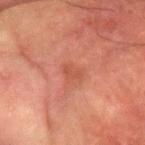Assessment: The lesion was photographed on a routine skin check and not biopsied; there is no pathology result. Context: The lesion is on the left forearm. Captured under cross-polarized illumination. A male subject roughly 80 years of age. The lesion-visualizer software estimated a mean CIELAB color near L≈41 a*≈24 b*≈27, about 5 CIELAB-L* units darker than the surrounding skin, and a lesion-to-skin contrast of about 5 (normalized; higher = more distinct). The analysis additionally found lesion-presence confidence of about 100/100. About 2.5 mm across. This image is a 15 mm lesion crop taken from a total-body photograph.